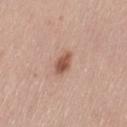Part of a total-body skin-imaging series; this lesion was reviewed on a skin check and was not flagged for biopsy.
A region of skin cropped from a whole-body photographic capture, roughly 15 mm wide.
The lesion's longest dimension is about 3 mm.
A female patient in their mid-60s.
Automated tile analysis of the lesion measured a lesion area of about 4.5 mm² and two-axis asymmetry of about 0.25. The software also gave a lesion color around L≈54 a*≈22 b*≈28 in CIELAB and a lesion-to-skin contrast of about 8.5 (normalized; higher = more distinct). And it measured an automated nevus-likeness rating near 95 out of 100.
On the left thigh.